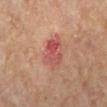follow-up — total-body-photography surveillance lesion; no biopsy | tile lighting — cross-polarized illumination | patient — female, aged 58 to 62 | TBP lesion metrics — a lesion area of about 10 mm², an eccentricity of roughly 0.6, and two-axis asymmetry of about 0.25; a border-irregularity rating of about 3/10 | image source — total-body-photography crop, ~15 mm field of view | site — the right lower leg.